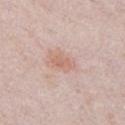This lesion was catalogued during total-body skin photography and was not selected for biopsy. A roughly 15 mm field-of-view crop from a total-body skin photograph. The lesion-visualizer software estimated about 8 CIELAB-L* units darker than the surrounding skin and a lesion-to-skin contrast of about 6 (normalized; higher = more distinct). And it measured a border-irregularity rating of about 3.5/10 and a color-variation rating of about 1.5/10. It also reported a nevus-likeness score of about 10/100. The lesion is located on the chest. Longest diameter approximately 3 mm. The tile uses white-light illumination. A male patient, in their mid-20s.